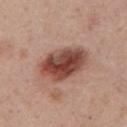This lesion was catalogued during total-body skin photography and was not selected for biopsy. The patient is a male aged around 75. A lesion tile, about 15 mm wide, cut from a 3D total-body photograph. On the front of the torso. The total-body-photography lesion software estimated a lesion color around L≈46 a*≈24 b*≈26 in CIELAB and roughly 17 lightness units darker than nearby skin. The software also gave an automated nevus-likeness rating near 95 out of 100. This is a white-light tile.